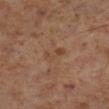<lesion>
  <biopsy_status>not biopsied; imaged during a skin examination</biopsy_status>
  <patient>
    <sex>male</sex>
    <age_approx>60</age_approx>
  </patient>
  <image>
    <source>total-body photography crop</source>
    <field_of_view_mm>15</field_of_view_mm>
  </image>
  <site>right lower leg</site>
  <lighting>cross-polarized</lighting>
  <lesion_size>
    <long_diameter_mm_approx>3.0</long_diameter_mm_approx>
  </lesion_size>
</lesion>